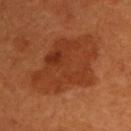The lesion was photographed on a routine skin check and not biopsied; there is no pathology result. Captured under cross-polarized illumination. Located on the back. A male subject about 60 years old. A roughly 15 mm field-of-view crop from a total-body skin photograph.